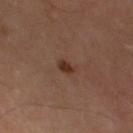- notes — catalogued during a skin exam; not biopsied
- subject — male, roughly 65 years of age
- imaging modality — ~15 mm crop, total-body skin-cancer survey
- site — the left thigh